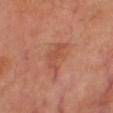Impression: Recorded during total-body skin imaging; not selected for excision or biopsy. Background: On the leg. A 15 mm close-up extracted from a 3D total-body photography capture. A male patient, about 70 years old. Approximately 4 mm at its widest. This is a cross-polarized tile.